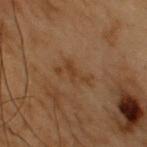The lesion was photographed on a routine skin check and not biopsied; there is no pathology result. A male patient, aged 53 to 57. A lesion tile, about 15 mm wide, cut from a 3D total-body photograph. The lesion is on the upper back.